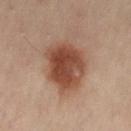Assessment:
Captured during whole-body skin photography for melanoma surveillance; the lesion was not biopsied.
Acquisition and patient details:
A male patient, roughly 50 years of age. A roughly 15 mm field-of-view crop from a total-body skin photograph. The lesion is located on the lower back.